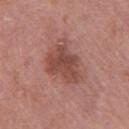Acquisition and patient details: On the right upper arm. A female patient aged 48–52. This image is a 15 mm lesion crop taken from a total-body photograph. An algorithmic analysis of the crop reported an area of roughly 15 mm², an outline eccentricity of about 0.75 (0 = round, 1 = elongated), and a shape-asymmetry score of about 0.3 (0 = symmetric). And it measured a mean CIELAB color near L≈47 a*≈24 b*≈25, roughly 10 lightness units darker than nearby skin, and a normalized lesion–skin contrast near 7.5. The analysis additionally found border irregularity of about 4 on a 0–10 scale and a color-variation rating of about 3.5/10. The software also gave a classifier nevus-likeness of about 10/100 and lesion-presence confidence of about 100/100.